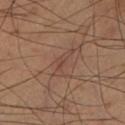{"biopsy_status": "not biopsied; imaged during a skin examination", "lighting": "cross-polarized", "patient": {"sex": "male", "age_approx": 60}, "site": "left lower leg", "image": {"source": "total-body photography crop", "field_of_view_mm": 15}, "lesion_size": {"long_diameter_mm_approx": 4.0}}